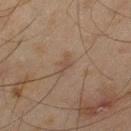Notes:
* biopsy status · total-body-photography surveillance lesion; no biopsy
* automated metrics · border irregularity of about 5.5 on a 0–10 scale, a color-variation rating of about 0/10, and a peripheral color-asymmetry measure near 0
* body site · the left thigh
* acquisition · ~15 mm crop, total-body skin-cancer survey
* patient · male, in their mid-40s
* illumination · cross-polarized illumination
* lesion size · ~2.5 mm (longest diameter)